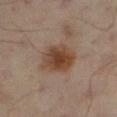<lesion>
  <lesion_size>
    <long_diameter_mm_approx>4.5</long_diameter_mm_approx>
  </lesion_size>
  <patient>
    <sex>male</sex>
    <age_approx>65</age_approx>
  </patient>
  <site>left lower leg</site>
  <lighting>cross-polarized</lighting>
  <image>
    <source>total-body photography crop</source>
    <field_of_view_mm>15</field_of_view_mm>
  </image>
</lesion>